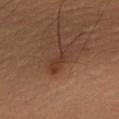The lesion was photographed on a routine skin check and not biopsied; there is no pathology result.
The subject is a male in their mid-30s.
The lesion is located on the arm.
A lesion tile, about 15 mm wide, cut from a 3D total-body photograph.
Longest diameter approximately 3.5 mm.
Automated image analysis of the tile measured an area of roughly 6 mm², a shape eccentricity near 0.85, and a shape-asymmetry score of about 0.35 (0 = symmetric). And it measured a mean CIELAB color near L≈32 a*≈18 b*≈25, about 6 CIELAB-L* units darker than the surrounding skin, and a lesion-to-skin contrast of about 5.5 (normalized; higher = more distinct). And it measured a nevus-likeness score of about 10/100.
This is a cross-polarized tile.